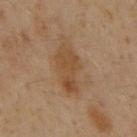The lesion was photographed on a routine skin check and not biopsied; there is no pathology result. On the mid back. A male subject, approximately 60 years of age. This is a cross-polarized tile. A 15 mm crop from a total-body photograph taken for skin-cancer surveillance. About 6 mm across. The lesion-visualizer software estimated an area of roughly 12 mm² and a symmetry-axis asymmetry near 0.25. The analysis additionally found an automated nevus-likeness rating near 25 out of 100 and a detector confidence of about 100 out of 100 that the crop contains a lesion.